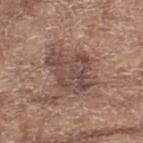Notes:
- biopsy status · imaged on a skin check; not biopsied
- body site · the leg
- automated lesion analysis · a mean CIELAB color near L≈47 a*≈17 b*≈22, a lesion–skin lightness drop of about 9, and a normalized lesion–skin contrast near 7.5; a border-irregularity rating of about 5/10, a within-lesion color-variation index near 4/10, and peripheral color asymmetry of about 1.5; a nevus-likeness score of about 0/100 and lesion-presence confidence of about 100/100
- diameter · ~5.5 mm (longest diameter)
- illumination · white-light
- subject · female, aged approximately 75
- image · 15 mm crop, total-body photography